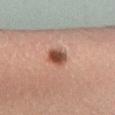Q: Is there a histopathology result?
A: imaged on a skin check; not biopsied
Q: How large is the lesion?
A: about 3 mm
Q: Patient demographics?
A: male, aged 18–22
Q: Lesion location?
A: the left lower leg
Q: How was this image acquired?
A: ~15 mm crop, total-body skin-cancer survey
Q: What lighting was used for the tile?
A: cross-polarized